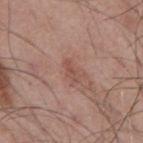biopsy status: total-body-photography surveillance lesion; no biopsy | image: 15 mm crop, total-body photography | body site: the back | TBP lesion metrics: a lesion area of about 2.5 mm², an outline eccentricity of about 0.9 (0 = round, 1 = elongated), and two-axis asymmetry of about 0.45; a nevus-likeness score of about 0/100 and a detector confidence of about 100 out of 100 that the crop contains a lesion | subject: male, in their mid-50s.